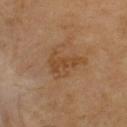Imaged during a routine full-body skin examination; the lesion was not biopsied and no histopathology is available.
A region of skin cropped from a whole-body photographic capture, roughly 15 mm wide.
Approximately 5 mm at its widest.
Located on the upper back.
The lesion-visualizer software estimated an area of roughly 9.5 mm² and a shape-asymmetry score of about 0.45 (0 = symmetric). It also reported a mean CIELAB color near L≈43 a*≈18 b*≈33 and a normalized lesion–skin contrast near 6. The software also gave a classifier nevus-likeness of about 0/100 and a lesion-detection confidence of about 100/100.
The subject is a male aged 68 to 72.
This is a cross-polarized tile.